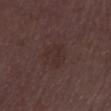biopsy status = catalogued during a skin exam; not biopsied | image = ~15 mm tile from a whole-body skin photo | size = about 3.5 mm | subject = female, aged 33 to 37 | illumination = white-light illumination | site = the left thigh.